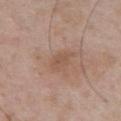Findings:
* biopsy status — total-body-photography surveillance lesion; no biopsy
* anatomic site — the chest
* patient — male, about 55 years old
* imaging modality — total-body-photography crop, ~15 mm field of view